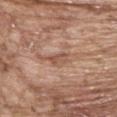This lesion was catalogued during total-body skin photography and was not selected for biopsy.
The lesion's longest dimension is about 2.5 mm.
Located on the head or neck.
A male patient roughly 65 years of age.
This is a white-light tile.
Cropped from a total-body skin-imaging series; the visible field is about 15 mm.